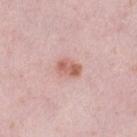Assessment:
The lesion was tiled from a total-body skin photograph and was not biopsied.
Clinical summary:
A roughly 15 mm field-of-view crop from a total-body skin photograph. About 3 mm across. Located on the left lower leg. Captured under white-light illumination. The patient is a female aged around 40.